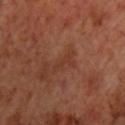Findings:
* follow-up · catalogued during a skin exam; not biopsied
* image · ~15 mm tile from a whole-body skin photo
* subject · male, roughly 70 years of age
* tile lighting · cross-polarized
* body site · the chest